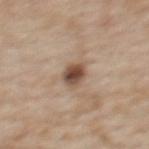Clinical impression: Captured during whole-body skin photography for melanoma surveillance; the lesion was not biopsied. Background: This image is a 15 mm lesion crop taken from a total-body photograph. Located on the upper back. A female subject, approximately 50 years of age. About 3 mm across. Imaged with white-light lighting.